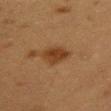Q: Is there a histopathology result?
A: no biopsy performed (imaged during a skin exam)
Q: Illumination type?
A: cross-polarized illumination
Q: What are the patient's age and sex?
A: female, aged approximately 40
Q: Lesion location?
A: the chest
Q: Automated lesion metrics?
A: an area of roughly 6.5 mm², a shape eccentricity near 0.7, and a symmetry-axis asymmetry near 0.2; a mean CIELAB color near L≈32 a*≈18 b*≈30; a border-irregularity index near 2/10, a within-lesion color-variation index near 2.5/10, and a peripheral color-asymmetry measure near 1
Q: How was this image acquired?
A: ~15 mm tile from a whole-body skin photo
Q: What is the lesion's diameter?
A: about 3.5 mm